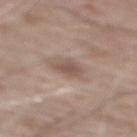| key | value |
|---|---|
| follow-up | catalogued during a skin exam; not biopsied |
| image source | ~15 mm crop, total-body skin-cancer survey |
| lighting | white-light |
| size | ≈3.5 mm |
| automated lesion analysis | a shape eccentricity near 0.65 and two-axis asymmetry of about 0.25; a classifier nevus-likeness of about 0/100 and a detector confidence of about 100 out of 100 that the crop contains a lesion |
| body site | the mid back |
| patient | male, in their 60s |